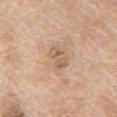notes: imaged on a skin check; not biopsied
body site: the chest
patient: male, in their mid- to late 60s
acquisition: ~15 mm crop, total-body skin-cancer survey
lighting: white-light illumination